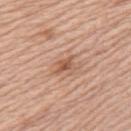The lesion was photographed on a routine skin check and not biopsied; there is no pathology result.
On the left upper arm.
A lesion tile, about 15 mm wide, cut from a 3D total-body photograph.
Longest diameter approximately 3 mm.
The subject is a female approximately 65 years of age.